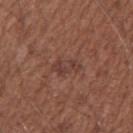Imaged during a routine full-body skin examination; the lesion was not biopsied and no histopathology is available. The recorded lesion diameter is about 3.5 mm. From the right upper arm. Imaged with white-light lighting. A male subject aged 53 to 57. Automated tile analysis of the lesion measured an area of roughly 5.5 mm² and two-axis asymmetry of about 0.35. And it measured a border-irregularity index near 4/10, internal color variation of about 3 on a 0–10 scale, and radial color variation of about 1. And it measured an automated nevus-likeness rating near 0 out of 100 and a detector confidence of about 100 out of 100 that the crop contains a lesion. A 15 mm crop from a total-body photograph taken for skin-cancer surveillance.